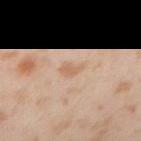Recorded during total-body skin imaging; not selected for excision or biopsy. This is a cross-polarized tile. On the right upper arm. Longest diameter approximately 2.5 mm. The subject is a male aged 53 to 57. A region of skin cropped from a whole-body photographic capture, roughly 15 mm wide.